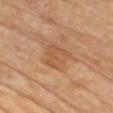follow-up — imaged on a skin check; not biopsied | automated metrics — internal color variation of about 2.5 on a 0–10 scale and a peripheral color-asymmetry measure near 1 | size — ~4 mm (longest diameter) | image source — total-body-photography crop, ~15 mm field of view | site — the chest | illumination — cross-polarized illumination | subject — female, in their mid-60s.